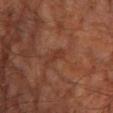• biopsy status: no biopsy performed (imaged during a skin exam)
• tile lighting: cross-polarized illumination
• patient: male, approximately 60 years of age
• image: 15 mm crop, total-body photography
• location: the leg
• diameter: ≈2.5 mm